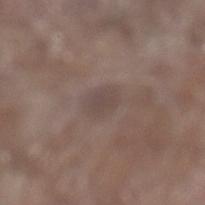Part of a total-body skin-imaging series; this lesion was reviewed on a skin check and was not flagged for biopsy. A lesion tile, about 15 mm wide, cut from a 3D total-body photograph. From the leg. The recorded lesion diameter is about 3 mm. Automated tile analysis of the lesion measured a lesion color around L≈45 a*≈13 b*≈19 in CIELAB, about 5 CIELAB-L* units darker than the surrounding skin, and a normalized border contrast of about 4.5. It also reported lesion-presence confidence of about 100/100. This is a white-light tile. The subject is a male aged 78 to 82.